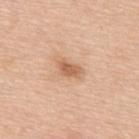Assessment:
The lesion was tiled from a total-body skin photograph and was not biopsied.
Background:
A male subject, aged around 50. About 3 mm across. Captured under white-light illumination. From the upper back. A roughly 15 mm field-of-view crop from a total-body skin photograph.